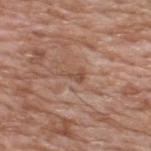| key | value |
|---|---|
| follow-up | catalogued during a skin exam; not biopsied |
| subject | male, about 60 years old |
| illumination | white-light illumination |
| lesion size | ≈2.5 mm |
| body site | the upper back |
| image-analysis metrics | a mean CIELAB color near L≈50 a*≈20 b*≈29 and roughly 7 lightness units darker than nearby skin; lesion-presence confidence of about 70/100 |
| image | ~15 mm crop, total-body skin-cancer survey |